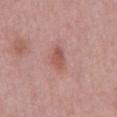Impression:
Part of a total-body skin-imaging series; this lesion was reviewed on a skin check and was not flagged for biopsy.
Clinical summary:
From the abdomen. A lesion tile, about 15 mm wide, cut from a 3D total-body photograph. The subject is a male approximately 50 years of age.